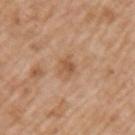- image: ~15 mm crop, total-body skin-cancer survey
- site: the left upper arm
- lesion diameter: ≈2.5 mm
- lighting: white-light
- patient: male, approximately 60 years of age